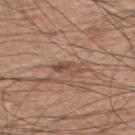Notes:
* location · the back
* subject · male, aged around 60
* imaging modality · 15 mm crop, total-body photography